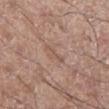| key | value |
|---|---|
| follow-up | no biopsy performed (imaged during a skin exam) |
| lesion diameter | about 3 mm |
| patient | male, about 60 years old |
| TBP lesion metrics | an average lesion color of about L≈54 a*≈18 b*≈26 (CIELAB) and a normalized border contrast of about 4.5 |
| lighting | white-light |
| body site | the right lower leg |
| imaging modality | total-body-photography crop, ~15 mm field of view |